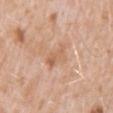The lesion is located on the mid back. The recorded lesion diameter is about 3.5 mm. A male patient about 50 years old. Cropped from a total-body skin-imaging series; the visible field is about 15 mm. This is a white-light tile.